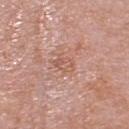biopsy status = no biopsy performed (imaged during a skin exam)
site = the head or neck
image = total-body-photography crop, ~15 mm field of view
patient = female, roughly 60 years of age